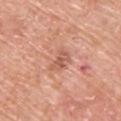Assessment: Recorded during total-body skin imaging; not selected for excision or biopsy. Background: An algorithmic analysis of the crop reported a footprint of about 4 mm², an eccentricity of roughly 0.75, and a symmetry-axis asymmetry near 0.35. A 15 mm crop from a total-body photograph taken for skin-cancer surveillance. A male patient aged around 60. From the upper back. The lesion's longest dimension is about 2.5 mm. The tile uses white-light illumination.